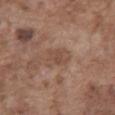Findings:
– notes — imaged on a skin check; not biopsied
– diameter — ≈3.5 mm
– acquisition — ~15 mm tile from a whole-body skin photo
– patient — male, in their mid- to late 70s
– tile lighting — white-light illumination
– body site — the abdomen